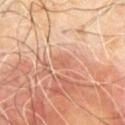biopsy_status: not biopsied; imaged during a skin examination
patient:
  sex: male
  age_approx: 60
lesion_size:
  long_diameter_mm_approx: 2.5
lighting: cross-polarized
site: upper back
image:
  source: total-body photography crop
  field_of_view_mm: 15